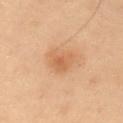follow-up=catalogued during a skin exam; not biopsied | size=~3.5 mm (longest diameter) | location=the abdomen | patient=male, in their mid- to late 50s | imaging modality=15 mm crop, total-body photography.